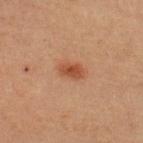Imaged during a routine full-body skin examination; the lesion was not biopsied and no histopathology is available. Captured under cross-polarized illumination. A region of skin cropped from a whole-body photographic capture, roughly 15 mm wide. Located on the upper back. The lesion-visualizer software estimated a lesion area of about 4.5 mm², an eccentricity of roughly 0.8, and a symmetry-axis asymmetry near 0.25. The software also gave a normalized lesion–skin contrast near 7.5. It also reported a border-irregularity index near 2.5/10, internal color variation of about 3 on a 0–10 scale, and peripheral color asymmetry of about 1. It also reported lesion-presence confidence of about 100/100. A male subject, in their 60s.